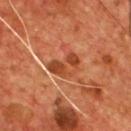Q: Was this lesion biopsied?
A: total-body-photography surveillance lesion; no biopsy
Q: Automated lesion metrics?
A: a footprint of about 8 mm² and a shape eccentricity near 0.75; a classifier nevus-likeness of about 0/100
Q: How large is the lesion?
A: ≈4 mm
Q: What are the patient's age and sex?
A: male, approximately 55 years of age
Q: How was this image acquired?
A: total-body-photography crop, ~15 mm field of view
Q: What lighting was used for the tile?
A: cross-polarized
Q: Where on the body is the lesion?
A: the chest